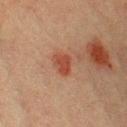The lesion was photographed on a routine skin check and not biopsied; there is no pathology result.
Automated image analysis of the tile measured a lesion area of about 4 mm² and a shape-asymmetry score of about 0.35 (0 = symmetric).
The lesion is located on the chest.
Longest diameter approximately 2.5 mm.
The patient is a male aged 38–42.
Cropped from a whole-body photographic skin survey; the tile spans about 15 mm.
Imaged with cross-polarized lighting.